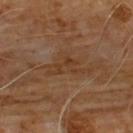follow-up: no biopsy performed (imaged during a skin exam)
automated metrics: a footprint of about 8.5 mm², a shape eccentricity near 0.8, and two-axis asymmetry of about 0.6; an average lesion color of about L≈36 a*≈18 b*≈30 (CIELAB), about 5 CIELAB-L* units darker than the surrounding skin, and a lesion-to-skin contrast of about 5.5 (normalized; higher = more distinct); a border-irregularity rating of about 8/10, internal color variation of about 2.5 on a 0–10 scale, and radial color variation of about 1
diameter: ≈5.5 mm
image: 15 mm crop, total-body photography
body site: the front of the torso
patient: male, in their 60s
tile lighting: cross-polarized